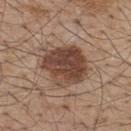{"biopsy_status": "not biopsied; imaged during a skin examination", "patient": {"sex": "male", "age_approx": 55}, "image": {"source": "total-body photography crop", "field_of_view_mm": 15}, "site": "upper back"}